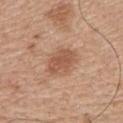  biopsy_status: not biopsied; imaged during a skin examination
  image:
    source: total-body photography crop
    field_of_view_mm: 15
  site: back
  lesion_size:
    long_diameter_mm_approx: 3.5
  patient:
    sex: male
    age_approx: 65
  lighting: white-light